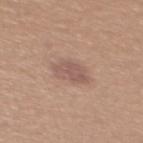Assessment: The lesion was tiled from a total-body skin photograph and was not biopsied.